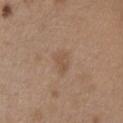This lesion was catalogued during total-body skin photography and was not selected for biopsy. A female subject approximately 40 years of age. The total-body-photography lesion software estimated an eccentricity of roughly 0.75 and a symmetry-axis asymmetry near 0.25. And it measured border irregularity of about 2.5 on a 0–10 scale and a within-lesion color-variation index near 2/10. It also reported a classifier nevus-likeness of about 0/100. Imaged with white-light lighting. This image is a 15 mm lesion crop taken from a total-body photograph. On the chest. Longest diameter approximately 3 mm.